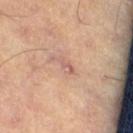  biopsy_status: not biopsied; imaged during a skin examination
  image:
    source: total-body photography crop
    field_of_view_mm: 15
  patient:
    sex: male
    age_approx: 60
  lesion_size:
    long_diameter_mm_approx: 2.5
  site: leg